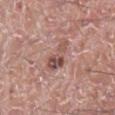| feature | finding |
|---|---|
| image | 15 mm crop, total-body photography |
| lesion diameter | about 4 mm |
| anatomic site | the right lower leg |
| tile lighting | white-light |
| patient | male, roughly 20 years of age |